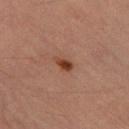Part of a total-body skin-imaging series; this lesion was reviewed on a skin check and was not flagged for biopsy. The subject is a female aged 68 to 72. Automated image analysis of the tile measured an average lesion color of about L≈36 a*≈21 b*≈27 (CIELAB) and about 10 CIELAB-L* units darker than the surrounding skin. On the left thigh. The tile uses cross-polarized illumination. A 15 mm close-up tile from a total-body photography series done for melanoma screening.